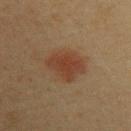Background: Captured under cross-polarized illumination. The subject is a female aged around 40. About 4 mm across. The total-body-photography lesion software estimated a mean CIELAB color near L≈34 a*≈18 b*≈26, about 7 CIELAB-L* units darker than the surrounding skin, and a lesion-to-skin contrast of about 7 (normalized; higher = more distinct). It also reported a border-irregularity index near 2.5/10, a color-variation rating of about 3/10, and radial color variation of about 1. It also reported a nevus-likeness score of about 95/100 and a detector confidence of about 100 out of 100 that the crop contains a lesion. On the left upper arm. A lesion tile, about 15 mm wide, cut from a 3D total-body photograph.